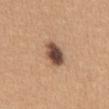This lesion was catalogued during total-body skin photography and was not selected for biopsy. Imaged with white-light lighting. Measured at roughly 3.5 mm in maximum diameter. A female patient aged approximately 35. This image is a 15 mm lesion crop taken from a total-body photograph. The lesion is located on the abdomen.